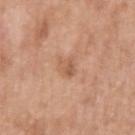Assessment:
The lesion was photographed on a routine skin check and not biopsied; there is no pathology result.
Image and clinical context:
A male subject, roughly 65 years of age. A 15 mm close-up tile from a total-body photography series done for melanoma screening. Captured under white-light illumination. The lesion-visualizer software estimated an area of roughly 4 mm² and a shape eccentricity near 0.65. The analysis additionally found a mean CIELAB color near L≈58 a*≈22 b*≈33, about 8 CIELAB-L* units darker than the surrounding skin, and a lesion-to-skin contrast of about 5.5 (normalized; higher = more distinct). The analysis additionally found a nevus-likeness score of about 0/100 and a lesion-detection confidence of about 100/100. Measured at roughly 2.5 mm in maximum diameter. The lesion is located on the right upper arm.